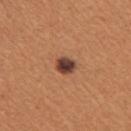{
  "biopsy_status": "not biopsied; imaged during a skin examination",
  "site": "right upper arm",
  "lesion_size": {
    "long_diameter_mm_approx": 2.5
  },
  "automated_metrics": {
    "area_mm2_approx": 4.5,
    "eccentricity": 0.55,
    "shape_asymmetry": 0.2,
    "cielab_L": 42,
    "cielab_a": 22,
    "cielab_b": 28,
    "vs_skin_darker_L": 16.0,
    "vs_skin_contrast_norm": 13.5
  },
  "lighting": "white-light",
  "patient": {
    "sex": "female",
    "age_approx": 40
  },
  "image": {
    "source": "total-body photography crop",
    "field_of_view_mm": 15
  }
}